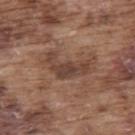notes: no biopsy performed (imaged during a skin exam) | size: ≈5 mm | image: ~15 mm tile from a whole-body skin photo | patient: male, aged around 75 | location: the upper back | illumination: white-light illumination | TBP lesion metrics: a mean CIELAB color near L≈42 a*≈18 b*≈25, a lesion–skin lightness drop of about 9, and a normalized border contrast of about 7.5; a border-irregularity rating of about 6.5/10, a within-lesion color-variation index near 3.5/10, and peripheral color asymmetry of about 1; a classifier nevus-likeness of about 0/100 and lesion-presence confidence of about 95/100.